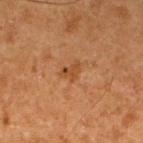| key | value |
|---|---|
| image source | total-body-photography crop, ~15 mm field of view |
| anatomic site | the upper back |
| subject | male, aged 63 to 67 |
| diameter | ≈2.5 mm |
| TBP lesion metrics | a nevus-likeness score of about 0/100 and a detector confidence of about 100 out of 100 that the crop contains a lesion |